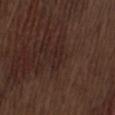Assessment:
Recorded during total-body skin imaging; not selected for excision or biopsy.
Acquisition and patient details:
A 15 mm crop from a total-body photograph taken for skin-cancer surveillance. On the lower back. The patient is a male roughly 70 years of age.